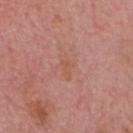Imaged during a routine full-body skin examination; the lesion was not biopsied and no histopathology is available.
A region of skin cropped from a whole-body photographic capture, roughly 15 mm wide.
Automated image analysis of the tile measured an eccentricity of roughly 0.75 and a symmetry-axis asymmetry near 0.3. The software also gave a mean CIELAB color near L≈55 a*≈23 b*≈30 and a normalized border contrast of about 5.
The patient is a male in their mid-70s.
About 3 mm across.
On the head or neck.
Imaged with white-light lighting.